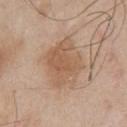This lesion was catalogued during total-body skin photography and was not selected for biopsy.
Captured under white-light illumination.
On the chest.
A male subject, in their mid- to late 60s.
Measured at roughly 5.5 mm in maximum diameter.
A roughly 15 mm field-of-view crop from a total-body skin photograph.